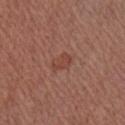The lesion was tiled from a total-body skin photograph and was not biopsied.
A male patient approximately 55 years of age.
About 2.5 mm across.
The lesion-visualizer software estimated a lesion area of about 3.5 mm², an outline eccentricity of about 0.75 (0 = round, 1 = elongated), and a symmetry-axis asymmetry near 0.25. The analysis additionally found a lesion color around L≈44 a*≈24 b*≈27 in CIELAB, a lesion–skin lightness drop of about 6, and a normalized border contrast of about 5.5. The software also gave a within-lesion color-variation index near 1/10 and peripheral color asymmetry of about 0.5.
Captured under white-light illumination.
A region of skin cropped from a whole-body photographic capture, roughly 15 mm wide.
The lesion is located on the right upper arm.